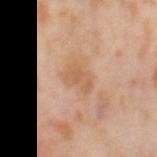Clinical impression: Captured during whole-body skin photography for melanoma surveillance; the lesion was not biopsied. Acquisition and patient details: Imaged with cross-polarized lighting. On the right thigh. A 15 mm close-up extracted from a 3D total-body photography capture. A female patient, roughly 55 years of age. Approximately 3 mm at its widest.